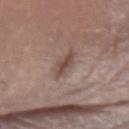The lesion was photographed on a routine skin check and not biopsied; there is no pathology result. A 15 mm crop from a total-body photograph taken for skin-cancer surveillance. The lesion is on the arm. A male patient about 65 years old.